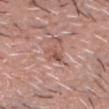<record>
  <biopsy_status>not biopsied; imaged during a skin examination</biopsy_status>
  <image>
    <source>total-body photography crop</source>
    <field_of_view_mm>15</field_of_view_mm>
  </image>
  <patient>
    <sex>male</sex>
    <age_approx>60</age_approx>
  </patient>
  <site>head or neck</site>
</record>